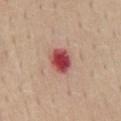notes = total-body-photography surveillance lesion; no biopsy
imaging modality = total-body-photography crop, ~15 mm field of view
tile lighting = white-light illumination
lesion diameter = ≈3 mm
location = the lower back
subject = female, aged around 50
image-analysis metrics = an average lesion color of about L≈48 a*≈35 b*≈24 (CIELAB), a lesion–skin lightness drop of about 18, and a normalized lesion–skin contrast near 12; a classifier nevus-likeness of about 0/100 and lesion-presence confidence of about 100/100